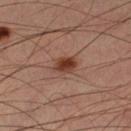Captured under cross-polarized illumination. From the left lower leg. Automated tile analysis of the lesion measured an outline eccentricity of about 0.6 (0 = round, 1 = elongated) and two-axis asymmetry of about 0.15. A male patient, roughly 45 years of age. A 15 mm close-up tile from a total-body photography series done for melanoma screening.